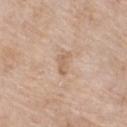| key | value |
|---|---|
| follow-up | no biopsy performed (imaged during a skin exam) |
| size | about 3 mm |
| anatomic site | the right thigh |
| subject | female, aged around 75 |
| image | total-body-photography crop, ~15 mm field of view |